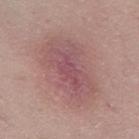Q: Was a biopsy performed?
A: total-body-photography surveillance lesion; no biopsy